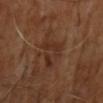Notes:
• follow-up: catalogued during a skin exam; not biopsied
• diameter: ≈6.5 mm
• image: ~15 mm crop, total-body skin-cancer survey
• illumination: cross-polarized illumination
• subject: male, aged around 65